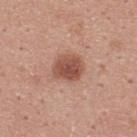This lesion was catalogued during total-body skin photography and was not selected for biopsy. An algorithmic analysis of the crop reported a footprint of about 9 mm², an eccentricity of roughly 0.55, and two-axis asymmetry of about 0.2. It also reported a mean CIELAB color near L≈51 a*≈24 b*≈28, a lesion–skin lightness drop of about 12, and a lesion-to-skin contrast of about 8.5 (normalized; higher = more distinct). The analysis additionally found an automated nevus-likeness rating near 95 out of 100 and a lesion-detection confidence of about 100/100. From the upper back. This image is a 15 mm lesion crop taken from a total-body photograph. Imaged with white-light lighting. The lesion's longest dimension is about 3.5 mm. A male patient in their mid-20s.